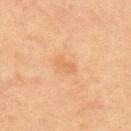Background:
The lesion-visualizer software estimated a border-irregularity rating of about 2.5/10, a color-variation rating of about 0/10, and a peripheral color-asymmetry measure near 0. Located on the chest. Imaged with cross-polarized lighting. The recorded lesion diameter is about 2.5 mm. A male subject, about 65 years old. A roughly 15 mm field-of-view crop from a total-body skin photograph.